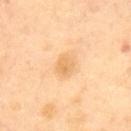  biopsy_status: not biopsied; imaged during a skin examination
  site: upper back
  patient:
    sex: male
    age_approx: 70
  image:
    source: total-body photography crop
    field_of_view_mm: 15